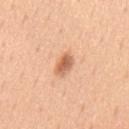Recorded during total-body skin imaging; not selected for excision or biopsy. The patient is a male in their 50s. A region of skin cropped from a whole-body photographic capture, roughly 15 mm wide. The tile uses white-light illumination. From the mid back.